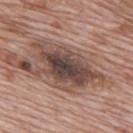Case summary:
• biopsy status — no biopsy performed (imaged during a skin exam)
• lesion size — about 8.5 mm
• TBP lesion metrics — a footprint of about 20 mm² and two-axis asymmetry of about 0.25
• patient — male, aged 68–72
• image source — total-body-photography crop, ~15 mm field of view
• tile lighting — white-light
• site — the upper back